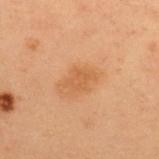Recorded during total-body skin imaging; not selected for excision or biopsy. A 15 mm close-up tile from a total-body photography series done for melanoma screening. From the upper back. A female subject aged 38–42.